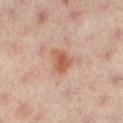Q: Was a biopsy performed?
A: no biopsy performed (imaged during a skin exam)
Q: Patient demographics?
A: female, approximately 40 years of age
Q: Automated lesion metrics?
A: a lesion area of about 6.5 mm² and a shape eccentricity near 0.6; a mean CIELAB color near L≈59 a*≈23 b*≈33, about 10 CIELAB-L* units darker than the surrounding skin, and a normalized lesion–skin contrast near 8; an automated nevus-likeness rating near 85 out of 100 and a detector confidence of about 100 out of 100 that the crop contains a lesion
Q: What is the imaging modality?
A: total-body-photography crop, ~15 mm field of view
Q: Where on the body is the lesion?
A: the right leg
Q: Illumination type?
A: cross-polarized